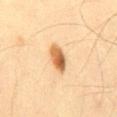The lesion was photographed on a routine skin check and not biopsied; there is no pathology result. The tile uses cross-polarized illumination. A male subject roughly 35 years of age. Cropped from a whole-body photographic skin survey; the tile spans about 15 mm. The lesion's longest dimension is about 4 mm. On the mid back.